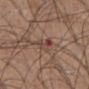Findings:
- notes: imaged on a skin check; not biopsied
- image-analysis metrics: a footprint of about 4.5 mm²; a lesion–skin lightness drop of about 8 and a lesion-to-skin contrast of about 7 (normalized; higher = more distinct); a color-variation rating of about 2.5/10 and peripheral color asymmetry of about 1
- location: the abdomen
- lesion size: about 3.5 mm
- tile lighting: white-light illumination
- acquisition: 15 mm crop, total-body photography
- subject: male, in their mid- to late 70s